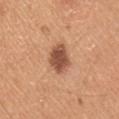biopsy status = total-body-photography surveillance lesion; no biopsy
imaging modality = ~15 mm tile from a whole-body skin photo
subject = male, about 20 years old
image-analysis metrics = an average lesion color of about L≈52 a*≈23 b*≈32 (CIELAB), about 15 CIELAB-L* units darker than the surrounding skin, and a normalized lesion–skin contrast near 10; border irregularity of about 2 on a 0–10 scale, internal color variation of about 3 on a 0–10 scale, and peripheral color asymmetry of about 1
anatomic site = the right upper arm